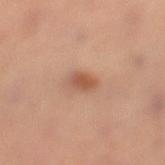Impression: This lesion was catalogued during total-body skin photography and was not selected for biopsy. Context: The lesion's longest dimension is about 3.5 mm. This is a cross-polarized tile. A 15 mm close-up tile from a total-body photography series done for melanoma screening. A male subject aged approximately 55. On the leg.